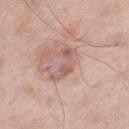{
  "biopsy_status": "not biopsied; imaged during a skin examination"
}